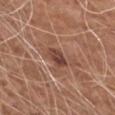Impression:
No biopsy was performed on this lesion — it was imaged during a full skin examination and was not determined to be concerning.
Image and clinical context:
Measured at roughly 3 mm in maximum diameter. A 15 mm close-up tile from a total-body photography series done for melanoma screening. Captured under white-light illumination. From the left upper arm. The patient is a male aged around 60.